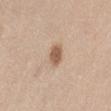biopsy status: no biopsy performed (imaged during a skin exam) | lesion diameter: about 3 mm | image source: total-body-photography crop, ~15 mm field of view | illumination: white-light | location: the left thigh | patient: female, aged around 40 | image-analysis metrics: a lesion area of about 4.5 mm², an outline eccentricity of about 0.75 (0 = round, 1 = elongated), and a shape-asymmetry score of about 0.15 (0 = symmetric); an average lesion color of about L≈58 a*≈18 b*≈31 (CIELAB) and a normalized lesion–skin contrast near 8.5; a border-irregularity index near 1.5/10, a within-lesion color-variation index near 2/10, and peripheral color asymmetry of about 0.5; an automated nevus-likeness rating near 95 out of 100 and a detector confidence of about 100 out of 100 that the crop contains a lesion.